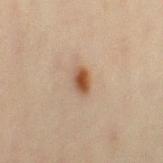  image:
    source: total-body photography crop
    field_of_view_mm: 15
  automated_metrics:
    area_mm2_approx: 4.0
    eccentricity: 0.8
    shape_asymmetry: 0.3
    border_irregularity_0_10: 2.5
    color_variation_0_10: 4.5
    peripheral_color_asymmetry: 1.5
    nevus_likeness_0_100: 100
    lesion_detection_confidence_0_100: 100
  lesion_size:
    long_diameter_mm_approx: 3.0
  site: right thigh
  lighting: cross-polarized
  patient:
    sex: female
    age_approx: 45